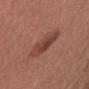Recorded during total-body skin imaging; not selected for excision or biopsy. The lesion is on the arm. A 15 mm close-up extracted from a 3D total-body photography capture. The tile uses white-light illumination. A female subject, aged around 50. The recorded lesion diameter is about 4 mm.